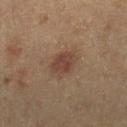Case summary:
– workup · catalogued during a skin exam; not biopsied
– anatomic site · the right lower leg
– subject · roughly 60 years of age
– illumination · cross-polarized
– size · about 3.5 mm
– automated lesion analysis · a footprint of about 8 mm² and an outline eccentricity of about 0.6 (0 = round, 1 = elongated); a lesion color around L≈42 a*≈18 b*≈26 in CIELAB, about 9 CIELAB-L* units darker than the surrounding skin, and a normalized border contrast of about 7.5; a border-irregularity rating of about 1.5/10, a color-variation rating of about 2.5/10, and a peripheral color-asymmetry measure near 1
– acquisition · ~15 mm tile from a whole-body skin photo